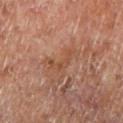No biopsy was performed on this lesion — it was imaged during a full skin examination and was not determined to be concerning. A 15 mm crop from a total-body photograph taken for skin-cancer surveillance. On the left lower leg. The subject is a male aged 68 to 72. Automated tile analysis of the lesion measured an area of roughly 4 mm² and a shape-asymmetry score of about 0.75 (0 = symmetric). The software also gave a lesion color around L≈47 a*≈22 b*≈31 in CIELAB, about 6 CIELAB-L* units darker than the surrounding skin, and a normalized border contrast of about 5.5. The software also gave border irregularity of about 7.5 on a 0–10 scale and radial color variation of about 0.